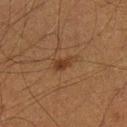Captured during whole-body skin photography for melanoma surveillance; the lesion was not biopsied. The lesion is located on the left lower leg. A 15 mm crop from a total-body photograph taken for skin-cancer surveillance. A male subject, approximately 40 years of age. The tile uses cross-polarized illumination.